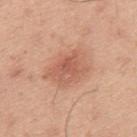<tbp_lesion>
  <biopsy_status>not biopsied; imaged during a skin examination</biopsy_status>
  <patient>
    <sex>male</sex>
    <age_approx>45</age_approx>
  </patient>
  <site>mid back</site>
  <image>
    <source>total-body photography crop</source>
    <field_of_view_mm>15</field_of_view_mm>
  </image>
</tbp_lesion>